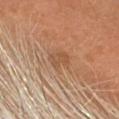Impression:
The lesion was photographed on a routine skin check and not biopsied; there is no pathology result.
Background:
Located on the head or neck. The recorded lesion diameter is about 3 mm. The tile uses cross-polarized illumination. A male subject, approximately 65 years of age. Cropped from a total-body skin-imaging series; the visible field is about 15 mm.